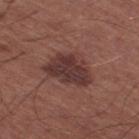| feature | finding |
|---|---|
| biopsy status | catalogued during a skin exam; not biopsied |
| subject | male, aged around 65 |
| imaging modality | 15 mm crop, total-body photography |
| location | the right thigh |